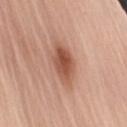No biopsy was performed on this lesion — it was imaged during a full skin examination and was not determined to be concerning. A 15 mm close-up extracted from a 3D total-body photography capture. A female subject, aged around 60. The lesion is on the arm. The recorded lesion diameter is about 4.5 mm.